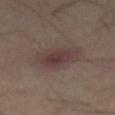Q: Was this lesion biopsied?
A: catalogued during a skin exam; not biopsied
Q: What kind of image is this?
A: ~15 mm tile from a whole-body skin photo
Q: What are the patient's age and sex?
A: male, roughly 50 years of age
Q: Lesion location?
A: the lower back
Q: What did automated image analysis measure?
A: an average lesion color of about L≈33 a*≈14 b*≈16 (CIELAB), a lesion–skin lightness drop of about 6, and a lesion-to-skin contrast of about 6.5 (normalized; higher = more distinct); a color-variation rating of about 4/10 and radial color variation of about 1.5; a nevus-likeness score of about 60/100 and a lesion-detection confidence of about 100/100
Q: Illumination type?
A: cross-polarized
Q: How large is the lesion?
A: about 4.5 mm